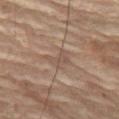Captured during whole-body skin photography for melanoma surveillance; the lesion was not biopsied. A female patient, aged around 80. On the right leg. The recorded lesion diameter is about 3 mm. This is a cross-polarized tile. A 15 mm crop from a total-body photograph taken for skin-cancer surveillance.